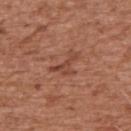Impression:
The lesion was tiled from a total-body skin photograph and was not biopsied.
Acquisition and patient details:
The patient is a male aged 73 to 77. A roughly 15 mm field-of-view crop from a total-body skin photograph. About 3.5 mm across. Located on the arm.